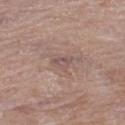The lesion was tiled from a total-body skin photograph and was not biopsied.
Cropped from a total-body skin-imaging series; the visible field is about 15 mm.
The subject is a female about 70 years old.
On the left thigh.